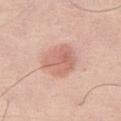Assessment: Part of a total-body skin-imaging series; this lesion was reviewed on a skin check and was not flagged for biopsy. Image and clinical context: From the right thigh. An algorithmic analysis of the crop reported a footprint of about 14 mm², an eccentricity of roughly 0.5, and a shape-asymmetry score of about 0.15 (0 = symmetric). It also reported a mean CIELAB color near L≈65 a*≈23 b*≈28. The software also gave a border-irregularity rating of about 1/10, internal color variation of about 4 on a 0–10 scale, and a peripheral color-asymmetry measure near 1.5. A male patient in their 70s. A region of skin cropped from a whole-body photographic capture, roughly 15 mm wide. Imaged with white-light lighting. The lesion's longest dimension is about 4.5 mm.